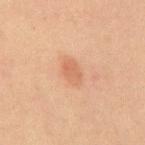Background:
Captured under cross-polarized illumination. Approximately 3 mm at its widest. A male patient, about 60 years old. The lesion is located on the mid back. Cropped from a total-body skin-imaging series; the visible field is about 15 mm.